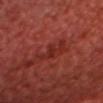| field | value |
|---|---|
| notes | imaged on a skin check; not biopsied |
| automated lesion analysis | a mean CIELAB color near L≈27 a*≈29 b*≈27, about 5 CIELAB-L* units darker than the surrounding skin, and a normalized lesion–skin contrast near 5.5; a classifier nevus-likeness of about 0/100 and a lesion-detection confidence of about 95/100 |
| lighting | cross-polarized |
| lesion size | about 2.5 mm |
| body site | the head or neck |
| patient | male, aged approximately 60 |
| image | ~15 mm tile from a whole-body skin photo |